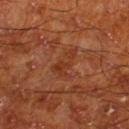Case summary:
– follow-up — catalogued during a skin exam; not biopsied
– automated lesion analysis — a lesion area of about 6.5 mm², an outline eccentricity of about 0.8 (0 = round, 1 = elongated), and a symmetry-axis asymmetry near 0.55
– patient — male, approximately 65 years of age
– image — ~15 mm crop, total-body skin-cancer survey
– location — the right lower leg
– lesion size — ~4 mm (longest diameter)
– tile lighting — cross-polarized